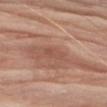Imaged during a routine full-body skin examination; the lesion was not biopsied and no histopathology is available.
A male subject, aged 78 to 82.
A lesion tile, about 15 mm wide, cut from a 3D total-body photograph.
From the right upper arm.